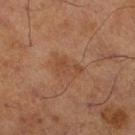Impression: The lesion was photographed on a routine skin check and not biopsied; there is no pathology result. Acquisition and patient details: A 15 mm close-up tile from a total-body photography series done for melanoma screening. A male subject aged around 70. On the right lower leg.